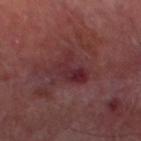biopsy_status: not biopsied; imaged during a skin examination
image:
  source: total-body photography crop
  field_of_view_mm: 15
patient:
  sex: male
  age_approx: 70
site: left thigh